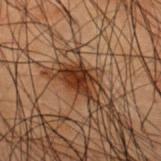Impression:
The lesion was photographed on a routine skin check and not biopsied; there is no pathology result.
Background:
The subject is a male about 50 years old. This is a cross-polarized tile. A 15 mm close-up tile from a total-body photography series done for melanoma screening. Longest diameter approximately 5 mm. The lesion is on the upper back.